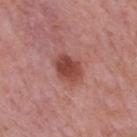No biopsy was performed on this lesion — it was imaged during a full skin examination and was not determined to be concerning.
A male patient aged around 75.
Cropped from a total-body skin-imaging series; the visible field is about 15 mm.
Located on the mid back.